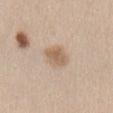notes = total-body-photography surveillance lesion; no biopsy
size = ≈3.5 mm
subject = female, in their 40s
automated lesion analysis = a footprint of about 7 mm² and an eccentricity of roughly 0.7
anatomic site = the abdomen
image = 15 mm crop, total-body photography